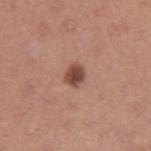Captured during whole-body skin photography for melanoma surveillance; the lesion was not biopsied.
Located on the left upper arm.
Automated image analysis of the tile measured a lesion area of about 4.5 mm², an eccentricity of roughly 0.55, and two-axis asymmetry of about 0.15. The analysis additionally found a border-irregularity rating of about 1.5/10, internal color variation of about 3.5 on a 0–10 scale, and a peripheral color-asymmetry measure near 1.
The subject is a male roughly 40 years of age.
A lesion tile, about 15 mm wide, cut from a 3D total-body photograph.
Imaged with white-light lighting.
About 2.5 mm across.